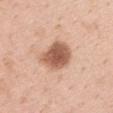| key | value |
|---|---|
| biopsy status | total-body-photography surveillance lesion; no biopsy |
| illumination | white-light |
| patient | female, aged 48 to 52 |
| acquisition | 15 mm crop, total-body photography |
| body site | the mid back |